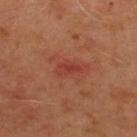workup = no biopsy performed (imaged during a skin exam)
subject = male, approximately 50 years of age
location = the upper back
imaging modality = ~15 mm crop, total-body skin-cancer survey
lighting = cross-polarized illumination
size = ~3 mm (longest diameter)